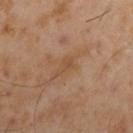The lesion was tiled from a total-body skin photograph and was not biopsied. The lesion is located on the left upper arm. The total-body-photography lesion software estimated a lesion area of about 2.5 mm², an eccentricity of roughly 0.85, and a shape-asymmetry score of about 0.45 (0 = symmetric). The analysis additionally found a mean CIELAB color near L≈49 a*≈19 b*≈33, roughly 6 lightness units darker than nearby skin, and a lesion-to-skin contrast of about 5 (normalized; higher = more distinct). A male subject, in their mid-50s. About 2.5 mm across. Cropped from a total-body skin-imaging series; the visible field is about 15 mm. Imaged with cross-polarized lighting.